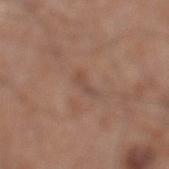{"biopsy_status": "not biopsied; imaged during a skin examination", "image": {"source": "total-body photography crop", "field_of_view_mm": 15}, "lesion_size": {"long_diameter_mm_approx": 3.0}, "site": "left lower leg", "lighting": "white-light", "automated_metrics": {"area_mm2_approx": 2.5, "shape_asymmetry": 0.45, "cielab_L": 48, "cielab_a": 19, "cielab_b": 26, "vs_skin_contrast_norm": 5.0, "nevus_likeness_0_100": 0, "lesion_detection_confidence_0_100": 95}, "patient": {"sex": "male", "age_approx": 20}}